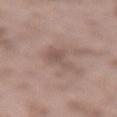A close-up tile cropped from a whole-body skin photograph, about 15 mm across.
The subject is a male in their mid- to late 50s.
The lesion is on the lower back.
Imaged with white-light lighting.